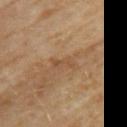Q: How was this image acquired?
A: ~15 mm crop, total-body skin-cancer survey
Q: What is the lesion's diameter?
A: ≈2.5 mm
Q: How was the tile lit?
A: cross-polarized illumination
Q: Patient demographics?
A: female, roughly 60 years of age
Q: What did automated image analysis measure?
A: an area of roughly 2 mm² and a shape eccentricity near 0.95; a lesion–skin lightness drop of about 7; border irregularity of about 6.5 on a 0–10 scale, a color-variation rating of about 0/10, and radial color variation of about 0; an automated nevus-likeness rating near 0 out of 100
Q: Lesion location?
A: the back